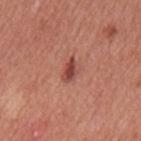workup — total-body-photography surveillance lesion; no biopsy
patient — male, aged around 45
image source — ~15 mm tile from a whole-body skin photo
anatomic site — the chest